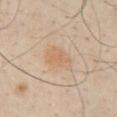Clinical impression: Imaged during a routine full-body skin examination; the lesion was not biopsied and no histopathology is available. Background: From the chest. Approximately 4 mm at its widest. Automated image analysis of the tile measured a lesion color around L≈66 a*≈17 b*≈33 in CIELAB and a lesion-to-skin contrast of about 5 (normalized; higher = more distinct). A male patient, aged 28–32. A close-up tile cropped from a whole-body skin photograph, about 15 mm across. Captured under white-light illumination.